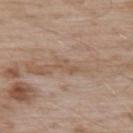Recorded during total-body skin imaging; not selected for excision or biopsy. A 15 mm close-up extracted from a 3D total-body photography capture. The subject is a male approximately 55 years of age. Located on the upper back.